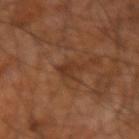notes: no biopsy performed (imaged during a skin exam) | diameter: ~3 mm (longest diameter) | imaging modality: 15 mm crop, total-body photography | anatomic site: the right forearm | patient: male, aged around 60 | image-analysis metrics: a lesion color around L≈33 a*≈21 b*≈30 in CIELAB and a lesion-to-skin contrast of about 6.5 (normalized; higher = more distinct); a border-irregularity index near 4/10; lesion-presence confidence of about 95/100 | lighting: cross-polarized illumination.